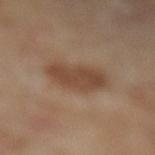notes: imaged on a skin check; not biopsied
subject: female, aged approximately 75
diameter: ≈5 mm
lighting: cross-polarized illumination
image-analysis metrics: a footprint of about 13 mm² and two-axis asymmetry of about 0.2; border irregularity of about 2.5 on a 0–10 scale and radial color variation of about 1
imaging modality: 15 mm crop, total-body photography
location: the right lower leg